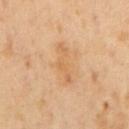Notes:
– patient: male, in their mid- to late 60s
– lighting: cross-polarized
– image: ~15 mm tile from a whole-body skin photo
– TBP lesion metrics: an area of roughly 6 mm², an outline eccentricity of about 0.95 (0 = round, 1 = elongated), and a shape-asymmetry score of about 0.5 (0 = symmetric); a lesion–skin lightness drop of about 7 and a normalized border contrast of about 5; border irregularity of about 7 on a 0–10 scale, internal color variation of about 1 on a 0–10 scale, and peripheral color asymmetry of about 0.5; a nevus-likeness score of about 0/100 and a lesion-detection confidence of about 100/100
– size: about 4.5 mm